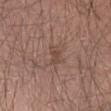Q: Was this lesion biopsied?
A: imaged on a skin check; not biopsied
Q: Where on the body is the lesion?
A: the left forearm
Q: What kind of image is this?
A: ~15 mm crop, total-body skin-cancer survey
Q: Patient demographics?
A: male, aged around 40